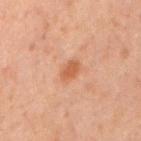Q: Was this lesion biopsied?
A: no biopsy performed (imaged during a skin exam)
Q: Where on the body is the lesion?
A: the mid back
Q: How was this image acquired?
A: ~15 mm crop, total-body skin-cancer survey
Q: What is the lesion's diameter?
A: ≈2.5 mm
Q: Automated lesion metrics?
A: an area of roughly 3.5 mm² and an eccentricity of roughly 0.8; a within-lesion color-variation index near 1/10
Q: Illumination type?
A: cross-polarized
Q: What are the patient's age and sex?
A: male, aged 68–72